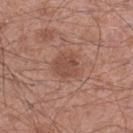Case summary:
- biopsy status · imaged on a skin check; not biopsied
- image source · ~15 mm crop, total-body skin-cancer survey
- lighting · white-light illumination
- patient · male, roughly 55 years of age
- body site · the left thigh
- lesion size · ~3 mm (longest diameter)